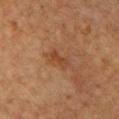Imaged during a routine full-body skin examination; the lesion was not biopsied and no histopathology is available.
On the chest.
This image is a 15 mm lesion crop taken from a total-body photograph.
The patient is a female about 50 years old.
The tile uses cross-polarized illumination.
Measured at roughly 2.5 mm in maximum diameter.
An algorithmic analysis of the crop reported a lesion color around L≈36 a*≈20 b*≈30 in CIELAB, roughly 6 lightness units darker than nearby skin, and a lesion-to-skin contrast of about 6 (normalized; higher = more distinct). It also reported border irregularity of about 3 on a 0–10 scale and peripheral color asymmetry of about 0.